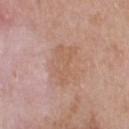Imaged during a routine full-body skin examination; the lesion was not biopsied and no histopathology is available. Measured at roughly 4.5 mm in maximum diameter. Captured under white-light illumination. A 15 mm crop from a total-body photograph taken for skin-cancer surveillance. A male subject in their mid-50s. From the upper back.